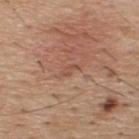biopsy status — total-body-photography surveillance lesion; no biopsy
acquisition — ~15 mm tile from a whole-body skin photo
body site — the upper back
subject — male, aged around 40
automated metrics — a footprint of about 2 mm² and two-axis asymmetry of about 0.45; a border-irregularity index near 4/10, a color-variation rating of about 0/10, and a peripheral color-asymmetry measure near 0; a nevus-likeness score of about 0/100